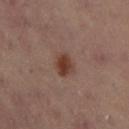Assessment: No biopsy was performed on this lesion — it was imaged during a full skin examination and was not determined to be concerning. Background: Measured at roughly 3 mm in maximum diameter. A lesion tile, about 15 mm wide, cut from a 3D total-body photograph. The total-body-photography lesion software estimated a shape eccentricity near 0.6 and two-axis asymmetry of about 0.2. The software also gave a border-irregularity index near 2/10, a color-variation rating of about 3/10, and radial color variation of about 1. Located on the left lower leg. The patient is a female in their mid-50s. The tile uses cross-polarized illumination.